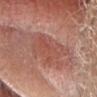Findings:
- workup · no biopsy performed (imaged during a skin exam)
- automated metrics · an outline eccentricity of about 0.8 (0 = round, 1 = elongated) and a shape-asymmetry score of about 0.4 (0 = symmetric); a lesion color around L≈51 a*≈26 b*≈28 in CIELAB, a lesion–skin lightness drop of about 7, and a normalized lesion–skin contrast near 5.5; border irregularity of about 6.5 on a 0–10 scale, internal color variation of about 2.5 on a 0–10 scale, and a peripheral color-asymmetry measure near 1
- lesion size · ~5.5 mm (longest diameter)
- image source · total-body-photography crop, ~15 mm field of view
- illumination · white-light illumination
- location · the head or neck
- subject · male, aged approximately 75